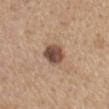<record>
<biopsy_status>not biopsied; imaged during a skin examination</biopsy_status>
</record>